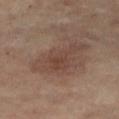The lesion was photographed on a routine skin check and not biopsied; there is no pathology result.
The patient is a female aged 63–67.
An algorithmic analysis of the crop reported an average lesion color of about L≈41 a*≈17 b*≈24 (CIELAB), roughly 7 lightness units darker than nearby skin, and a lesion-to-skin contrast of about 6.5 (normalized; higher = more distinct). It also reported border irregularity of about 5 on a 0–10 scale, internal color variation of about 3 on a 0–10 scale, and a peripheral color-asymmetry measure near 1.
Captured under cross-polarized illumination.
About 7.5 mm across.
On the leg.
A lesion tile, about 15 mm wide, cut from a 3D total-body photograph.